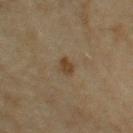- workup: no biopsy performed (imaged during a skin exam)
- imaging modality: ~15 mm tile from a whole-body skin photo
- automated metrics: a footprint of about 3 mm² and two-axis asymmetry of about 0.25; a nevus-likeness score of about 70/100
- patient: female, roughly 60 years of age
- anatomic site: the right upper arm
- lighting: cross-polarized illumination